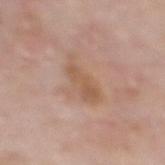Impression: The lesion was photographed on a routine skin check and not biopsied; there is no pathology result. Background: Located on the upper back. Cropped from a whole-body photographic skin survey; the tile spans about 15 mm. The total-body-photography lesion software estimated a mean CIELAB color near L≈56 a*≈18 b*≈31, about 7 CIELAB-L* units darker than the surrounding skin, and a normalized lesion–skin contrast near 6.5. A female subject, roughly 50 years of age.